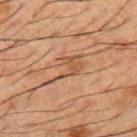| key | value |
|---|---|
| notes | total-body-photography surveillance lesion; no biopsy |
| patient | male, aged around 50 |
| anatomic site | the mid back |
| lesion diameter | about 5 mm |
| image source | 15 mm crop, total-body photography |